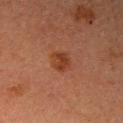Q: Is there a histopathology result?
A: catalogued during a skin exam; not biopsied
Q: How was the tile lit?
A: cross-polarized
Q: Automated lesion metrics?
A: a footprint of about 5.5 mm²; a mean CIELAB color near L≈32 a*≈21 b*≈28, about 7 CIELAB-L* units darker than the surrounding skin, and a normalized lesion–skin contrast near 7.5; a color-variation rating of about 3/10 and peripheral color asymmetry of about 1; a nevus-likeness score of about 90/100 and a detector confidence of about 100 out of 100 that the crop contains a lesion
Q: Where on the body is the lesion?
A: the right upper arm
Q: What are the patient's age and sex?
A: female, roughly 60 years of age
Q: How was this image acquired?
A: ~15 mm crop, total-body skin-cancer survey
Q: Lesion size?
A: ≈2.5 mm